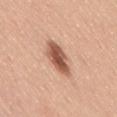| field | value |
|---|---|
| follow-up | imaged on a skin check; not biopsied |
| patient | male, in their 50s |
| anatomic site | the lower back |
| tile lighting | white-light illumination |
| image | ~15 mm crop, total-body skin-cancer survey |
| size | ≈5 mm |